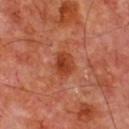The lesion was photographed on a routine skin check and not biopsied; there is no pathology result. A male subject, aged approximately 70. A close-up tile cropped from a whole-body skin photograph, about 15 mm across. Automated tile analysis of the lesion measured a shape eccentricity near 0.65 and a symmetry-axis asymmetry near 0.15. The analysis additionally found a mean CIELAB color near L≈42 a*≈32 b*≈37 and a lesion–skin lightness drop of about 10. It also reported a border-irregularity rating of about 1.5/10, a color-variation rating of about 3.5/10, and radial color variation of about 1.5. The analysis additionally found a classifier nevus-likeness of about 75/100 and a lesion-detection confidence of about 100/100. This is a cross-polarized tile. From the front of the torso.